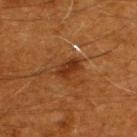workup: catalogued during a skin exam; not biopsied | site: the upper back | lesion diameter: about 3 mm | subject: male, aged 58–62 | TBP lesion metrics: a lesion area of about 4.5 mm² and two-axis asymmetry of about 0.25; a lesion-detection confidence of about 100/100 | illumination: cross-polarized | image source: ~15 mm tile from a whole-body skin photo.